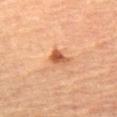This lesion was catalogued during total-body skin photography and was not selected for biopsy. A male subject, in their mid- to late 60s. Captured under cross-polarized illumination. The lesion is located on the right thigh. This image is a 15 mm lesion crop taken from a total-body photograph.